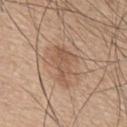anatomic site: the mid back
lighting: white-light illumination
size: ≈5.5 mm
patient: male, aged around 65
automated lesion analysis: a footprint of about 8.5 mm², an outline eccentricity of about 0.85 (0 = round, 1 = elongated), and two-axis asymmetry of about 0.45; border irregularity of about 6 on a 0–10 scale, a color-variation rating of about 2.5/10, and a peripheral color-asymmetry measure near 1; a lesion-detection confidence of about 100/100
imaging modality: 15 mm crop, total-body photography